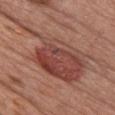biopsy status=catalogued during a skin exam; not biopsied
acquisition=~15 mm crop, total-body skin-cancer survey
subject=female, aged 58–62
TBP lesion metrics=a lesion color around L≈45 a*≈25 b*≈26 in CIELAB, about 10 CIELAB-L* units darker than the surrounding skin, and a normalized border contrast of about 8; a nevus-likeness score of about 5/100 and a detector confidence of about 95 out of 100 that the crop contains a lesion
anatomic site=the chest
size=≈11.5 mm
illumination=white-light illumination